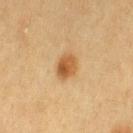biopsy status: imaged on a skin check; not biopsied
lighting: cross-polarized illumination
automated metrics: an area of roughly 6.5 mm², an eccentricity of roughly 0.75, and two-axis asymmetry of about 0.2; a mean CIELAB color near L≈47 a*≈18 b*≈36, about 11 CIELAB-L* units darker than the surrounding skin, and a lesion-to-skin contrast of about 8.5 (normalized; higher = more distinct); a nevus-likeness score of about 100/100 and lesion-presence confidence of about 100/100
subject: female, aged around 55
image: ~15 mm crop, total-body skin-cancer survey
location: the left thigh
size: about 3.5 mm